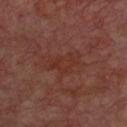Case summary:
- notes: imaged on a skin check; not biopsied
- image: ~15 mm tile from a whole-body skin photo
- lesion diameter: about 3.5 mm
- subject: male, approximately 65 years of age
- lighting: cross-polarized
- body site: the chest